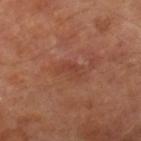Clinical impression: The lesion was tiled from a total-body skin photograph and was not biopsied. Background: A male subject, about 70 years old. Measured at roughly 3 mm in maximum diameter. The lesion is on the right lower leg. This is a cross-polarized tile. A 15 mm close-up extracted from a 3D total-body photography capture.